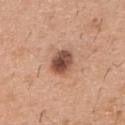<tbp_lesion>
  <biopsy_status>not biopsied; imaged during a skin examination</biopsy_status>
</tbp_lesion>